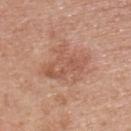Findings:
– notes — no biopsy performed (imaged during a skin exam)
– patient — female, aged around 60
– lesion size — ≈5 mm
– automated lesion analysis — a mean CIELAB color near L≈56 a*≈23 b*≈30 and roughly 8 lightness units darker than nearby skin; a border-irregularity index near 6/10, internal color variation of about 4 on a 0–10 scale, and peripheral color asymmetry of about 1; an automated nevus-likeness rating near 0 out of 100 and a detector confidence of about 100 out of 100 that the crop contains a lesion
– site — the left upper arm
– image source — 15 mm crop, total-body photography